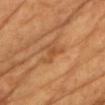Imaged during a routine full-body skin examination; the lesion was not biopsied and no histopathology is available.
On the chest.
A 15 mm close-up extracted from a 3D total-body photography capture.
A female patient aged 68–72.
Automated tile analysis of the lesion measured a shape eccentricity near 0.7 and a shape-asymmetry score of about 0.6 (0 = symmetric). The software also gave a lesion color around L≈49 a*≈23 b*≈38 in CIELAB and about 7 CIELAB-L* units darker than the surrounding skin.
This is a cross-polarized tile.
Approximately 3.5 mm at its widest.